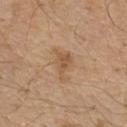follow-up: imaged on a skin check; not biopsied | size: ≈4 mm | illumination: white-light illumination | subject: male, aged 68 to 72 | image source: total-body-photography crop, ~15 mm field of view | image-analysis metrics: an area of roughly 7 mm², an outline eccentricity of about 0.8 (0 = round, 1 = elongated), and a shape-asymmetry score of about 0.45 (0 = symmetric) | location: the front of the torso.